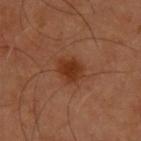follow-up: imaged on a skin check; not biopsied
lighting: cross-polarized illumination
image source: ~15 mm crop, total-body skin-cancer survey
diameter: about 3.5 mm
TBP lesion metrics: a border-irregularity index near 2.5/10 and a within-lesion color-variation index near 2/10; a nevus-likeness score of about 95/100 and a lesion-detection confidence of about 100/100
anatomic site: the back
subject: male, approximately 55 years of age